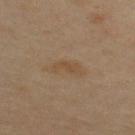biopsy_status: not biopsied; imaged during a skin examination
site: upper back
lesion_size:
  long_diameter_mm_approx: 3.5
image:
  source: total-body photography crop
  field_of_view_mm: 15
patient:
  sex: male
  age_approx: 35